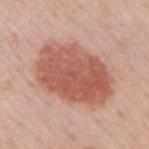Q: Illumination type?
A: white-light
Q: What did automated image analysis measure?
A: an eccentricity of roughly 0.75 and a shape-asymmetry score of about 0.1 (0 = symmetric)
Q: What are the patient's age and sex?
A: male, approximately 60 years of age
Q: Where on the body is the lesion?
A: the right upper arm
Q: What is the imaging modality?
A: ~15 mm crop, total-body skin-cancer survey
Q: Lesion size?
A: ~8.5 mm (longest diameter)